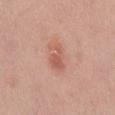Assessment: Captured during whole-body skin photography for melanoma surveillance; the lesion was not biopsied. Background: A roughly 15 mm field-of-view crop from a total-body skin photograph. Approximately 4 mm at its widest. The lesion is on the mid back. The tile uses white-light illumination. A male patient approximately 35 years of age.